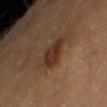workup — total-body-photography surveillance lesion; no biopsy | location — the right upper arm | tile lighting — cross-polarized illumination | subject — female, aged 53 to 57 | size — ≈3.5 mm | image — ~15 mm tile from a whole-body skin photo.